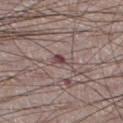The lesion was tiled from a total-body skin photograph and was not biopsied. A male patient roughly 75 years of age. About 2.5 mm across. Located on the leg. A region of skin cropped from a whole-body photographic capture, roughly 15 mm wide.